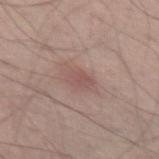The lesion was tiled from a total-body skin photograph and was not biopsied. The lesion is on the left thigh. The subject is a male approximately 55 years of age. A close-up tile cropped from a whole-body skin photograph, about 15 mm across.